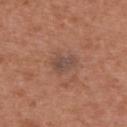workup = imaged on a skin check; not biopsied.